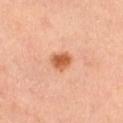workup: catalogued during a skin exam; not biopsied
subject: female, aged 33 to 37
site: the leg
acquisition: 15 mm crop, total-body photography